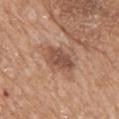Q: Was a biopsy performed?
A: catalogued during a skin exam; not biopsied
Q: What is the imaging modality?
A: 15 mm crop, total-body photography
Q: How was the tile lit?
A: white-light illumination
Q: What is the lesion's diameter?
A: ≈4 mm
Q: Automated lesion metrics?
A: a lesion color around L≈51 a*≈21 b*≈29 in CIELAB and a normalized border contrast of about 7.5; border irregularity of about 2.5 on a 0–10 scale and a within-lesion color-variation index near 3/10
Q: Who is the patient?
A: female, roughly 70 years of age
Q: Lesion location?
A: the mid back